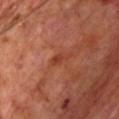Context:
A region of skin cropped from a whole-body photographic capture, roughly 15 mm wide. About 2.5 mm across. The patient is a male roughly 70 years of age. Imaged with cross-polarized lighting. On the chest.